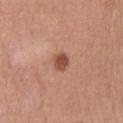Imaged during a routine full-body skin examination; the lesion was not biopsied and no histopathology is available.
Measured at roughly 2.5 mm in maximum diameter.
The lesion is on the right upper arm.
A female subject, aged 58 to 62.
A lesion tile, about 15 mm wide, cut from a 3D total-body photograph.